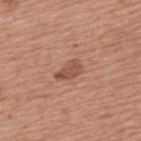{"biopsy_status": "not biopsied; imaged during a skin examination", "lesion_size": {"long_diameter_mm_approx": 3.0}, "image": {"source": "total-body photography crop", "field_of_view_mm": 15}, "site": "upper back", "lighting": "white-light", "patient": {"sex": "male", "age_approx": 75}, "automated_metrics": {"area_mm2_approx": 4.5, "eccentricity": 0.85, "shape_asymmetry": 0.3, "vs_skin_darker_L": 10.0}}